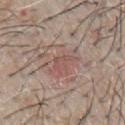Q: Is there a histopathology result?
A: total-body-photography surveillance lesion; no biopsy
Q: What is the imaging modality?
A: ~15 mm crop, total-body skin-cancer survey
Q: Illumination type?
A: white-light illumination
Q: What is the anatomic site?
A: the chest
Q: Patient demographics?
A: male, aged 63–67
Q: Lesion size?
A: ~3 mm (longest diameter)
Q: What did automated image analysis measure?
A: a lesion color around L≈52 a*≈20 b*≈23 in CIELAB, a lesion–skin lightness drop of about 6, and a normalized lesion–skin contrast near 4.5; a color-variation rating of about 1/10; a nevus-likeness score of about 50/100 and lesion-presence confidence of about 60/100